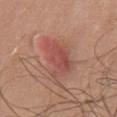Part of a total-body skin-imaging series; this lesion was reviewed on a skin check and was not flagged for biopsy. A male patient, about 70 years old. A lesion tile, about 15 mm wide, cut from a 3D total-body photograph. Longest diameter approximately 6 mm. Automated image analysis of the tile measured a shape eccentricity near 0.9 and a shape-asymmetry score of about 0.35 (0 = symmetric). The lesion is on the front of the torso.